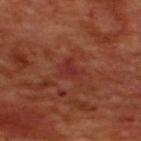subject: male, aged 48–52 | image: ~15 mm tile from a whole-body skin photo | illumination: cross-polarized | automated lesion analysis: a mean CIELAB color near L≈33 a*≈31 b*≈27, a lesion–skin lightness drop of about 5, and a normalized lesion–skin contrast near 5.5 | diameter: ≈3 mm | site: the upper back.